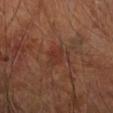Clinical impression:
No biopsy was performed on this lesion — it was imaged during a full skin examination and was not determined to be concerning.
Clinical summary:
The patient is a male in their mid- to late 60s. On the arm. Cropped from a whole-body photographic skin survey; the tile spans about 15 mm.